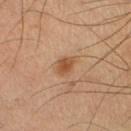Q: Was this lesion biopsied?
A: total-body-photography surveillance lesion; no biopsy
Q: What are the patient's age and sex?
A: male, aged 38 to 42
Q: What kind of image is this?
A: ~15 mm crop, total-body skin-cancer survey
Q: Lesion size?
A: ≈2.5 mm
Q: What is the anatomic site?
A: the right lower leg
Q: What lighting was used for the tile?
A: cross-polarized illumination